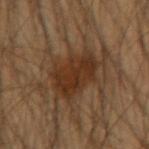Imaged during a routine full-body skin examination; the lesion was not biopsied and no histopathology is available.
From the right upper arm.
Longest diameter approximately 5 mm.
The tile uses cross-polarized illumination.
A male subject, aged around 45.
A roughly 15 mm field-of-view crop from a total-body skin photograph.